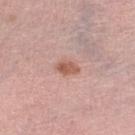Assessment: Recorded during total-body skin imaging; not selected for excision or biopsy. Image and clinical context: A roughly 15 mm field-of-view crop from a total-body skin photograph. On the left lower leg. Captured under white-light illumination. The patient is a female in their mid- to late 60s. Automated tile analysis of the lesion measured a lesion color around L≈57 a*≈24 b*≈28 in CIELAB, a lesion–skin lightness drop of about 11, and a normalized border contrast of about 8.